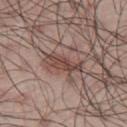<tbp_lesion>
  <biopsy_status>not biopsied; imaged during a skin examination</biopsy_status>
  <image>
    <source>total-body photography crop</source>
    <field_of_view_mm>15</field_of_view_mm>
  </image>
  <automated_metrics>
    <area_mm2_approx>7.0</area_mm2_approx>
    <eccentricity>0.95</eccentricity>
    <shape_asymmetry>0.3</shape_asymmetry>
    <cielab_L>45</cielab_L>
    <cielab_a>19</cielab_a>
    <cielab_b>22</cielab_b>
    <vs_skin_darker_L>11.0</vs_skin_darker_L>
    <nevus_likeness_0_100>90</nevus_likeness_0_100>
    <lesion_detection_confidence_0_100>100</lesion_detection_confidence_0_100>
  </automated_metrics>
  <lesion_size>
    <long_diameter_mm_approx>4.5</long_diameter_mm_approx>
  </lesion_size>
  <patient>
    <sex>male</sex>
    <age_approx>45</age_approx>
  </patient>
  <site>upper back</site>
</tbp_lesion>